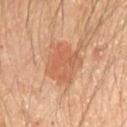Findings:
• biopsy status — no biopsy performed (imaged during a skin exam)
• body site — the right upper arm
• lighting — cross-polarized
• diameter — ~5 mm (longest diameter)
• image source — total-body-photography crop, ~15 mm field of view
• automated lesion analysis — an area of roughly 16 mm², a shape eccentricity near 0.6, and two-axis asymmetry of about 0.2; a border-irregularity rating of about 3.5/10 and a color-variation rating of about 4/10
• subject — male, approximately 65 years of age